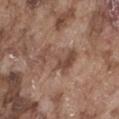<tbp_lesion>
<image>
  <source>total-body photography crop</source>
  <field_of_view_mm>15</field_of_view_mm>
</image>
<site>abdomen</site>
<lesion_size>
  <long_diameter_mm_approx>5.0</long_diameter_mm_approx>
</lesion_size>
<patient>
  <sex>male</sex>
  <age_approx>75</age_approx>
</patient>
</tbp_lesion>